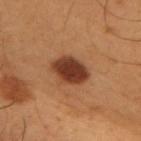Captured during whole-body skin photography for melanoma surveillance; the lesion was not biopsied. Automated tile analysis of the lesion measured an eccentricity of roughly 0.7 and a symmetry-axis asymmetry near 0.15. The analysis additionally found an average lesion color of about L≈37 a*≈24 b*≈31 (CIELAB), about 15 CIELAB-L* units darker than the surrounding skin, and a normalized border contrast of about 12.5. The analysis additionally found a classifier nevus-likeness of about 100/100. A male patient, in their mid- to late 50s. On the upper back. Approximately 4.5 mm at its widest. This is a cross-polarized tile. Cropped from a total-body skin-imaging series; the visible field is about 15 mm.